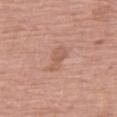{
  "biopsy_status": "not biopsied; imaged during a skin examination",
  "image": {
    "source": "total-body photography crop",
    "field_of_view_mm": 15
  },
  "site": "right thigh",
  "patient": {
    "sex": "female",
    "age_approx": 65
  }
}